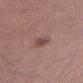The lesion was photographed on a routine skin check and not biopsied; there is no pathology result. The lesion-visualizer software estimated a mean CIELAB color near L≈48 a*≈20 b*≈21 and a normalized lesion–skin contrast near 6.5. And it measured a border-irregularity rating of about 2.5/10, internal color variation of about 3 on a 0–10 scale, and a peripheral color-asymmetry measure near 1. The software also gave an automated nevus-likeness rating near 30 out of 100 and a detector confidence of about 100 out of 100 that the crop contains a lesion. The recorded lesion diameter is about 3 mm. Located on the leg. A male subject, aged 73 to 77. The tile uses white-light illumination. A 15 mm close-up tile from a total-body photography series done for melanoma screening.